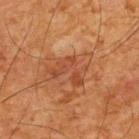The lesion was tiled from a total-body skin photograph and was not biopsied.
Approximately 5.5 mm at its widest.
A roughly 15 mm field-of-view crop from a total-body skin photograph.
The subject is a male in their mid- to late 60s.
From the back.
The tile uses cross-polarized illumination.